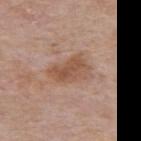Recorded during total-body skin imaging; not selected for excision or biopsy. An algorithmic analysis of the crop reported a border-irregularity index near 3/10, a color-variation rating of about 4/10, and a peripheral color-asymmetry measure near 1.5. About 5 mm across. A 15 mm close-up tile from a total-body photography series done for melanoma screening. The tile uses white-light illumination. The lesion is located on the mid back. A male subject, aged 73 to 77.